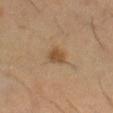Background: Imaged with cross-polarized lighting. Automated tile analysis of the lesion measured a lesion area of about 4 mm², an outline eccentricity of about 0.6 (0 = round, 1 = elongated), and two-axis asymmetry of about 0.25. It also reported an average lesion color of about L≈46 a*≈17 b*≈32 (CIELAB) and a normalized border contrast of about 7.5. And it measured a border-irregularity index near 2/10. The software also gave a classifier nevus-likeness of about 85/100 and a detector confidence of about 100 out of 100 that the crop contains a lesion. Located on the left thigh. A male patient, roughly 60 years of age. A region of skin cropped from a whole-body photographic capture, roughly 15 mm wide. Measured at roughly 2.5 mm in maximum diameter.